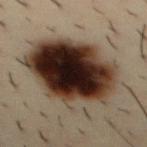{"biopsy_status": "not biopsied; imaged during a skin examination", "lighting": "cross-polarized", "patient": {"sex": "male", "age_approx": 30}, "site": "abdomen", "image": {"source": "total-body photography crop", "field_of_view_mm": 15}, "lesion_size": {"long_diameter_mm_approx": 10.0}, "automated_metrics": {"eccentricity": 0.55, "shape_asymmetry": 0.2}}